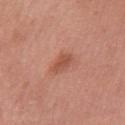follow-up = total-body-photography surveillance lesion; no biopsy | subject = female, about 70 years old | image source = ~15 mm tile from a whole-body skin photo | size = ≈3 mm | body site = the chest | automated metrics = an automated nevus-likeness rating near 65 out of 100 and lesion-presence confidence of about 100/100.